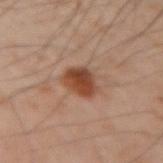Imaged during a routine full-body skin examination; the lesion was not biopsied and no histopathology is available. A region of skin cropped from a whole-body photographic capture, roughly 15 mm wide. A male patient aged 48 to 52. Imaged with cross-polarized lighting. The lesion's longest dimension is about 3 mm. From the left arm.